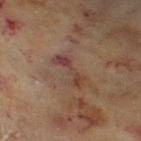Part of a total-body skin-imaging series; this lesion was reviewed on a skin check and was not flagged for biopsy.
The subject is a female roughly 60 years of age.
The lesion is located on the left lower leg.
About 4.5 mm across.
Cropped from a whole-body photographic skin survey; the tile spans about 15 mm.